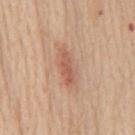follow-up — imaged on a skin check; not biopsied
anatomic site — the mid back
size — ~4.5 mm (longest diameter)
automated metrics — about 10 CIELAB-L* units darker than the surrounding skin and a lesion-to-skin contrast of about 6.5 (normalized; higher = more distinct); a within-lesion color-variation index near 3/10 and a peripheral color-asymmetry measure near 1; a classifier nevus-likeness of about 60/100
patient — male, aged 58 to 62
acquisition — ~15 mm tile from a whole-body skin photo